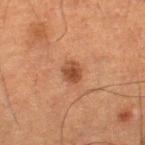This lesion was catalogued during total-body skin photography and was not selected for biopsy. The lesion is located on the right lower leg. A 15 mm close-up extracted from a 3D total-body photography capture. A male patient roughly 75 years of age.